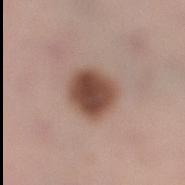Clinical impression:
This lesion was catalogued during total-body skin photography and was not selected for biopsy.
Background:
The lesion is located on the right thigh. A 15 mm close-up tile from a total-body photography series done for melanoma screening. A female patient aged around 30. This is a white-light tile. About 4.5 mm across.